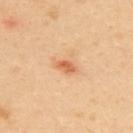| field | value |
|---|---|
| follow-up | catalogued during a skin exam; not biopsied |